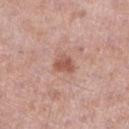Assessment: No biopsy was performed on this lesion — it was imaged during a full skin examination and was not determined to be concerning. Context: The tile uses white-light illumination. On the right lower leg. A male subject aged around 75. Approximately 2.5 mm at its widest. A roughly 15 mm field-of-view crop from a total-body skin photograph.